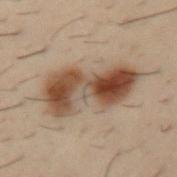follow-up: total-body-photography surveillance lesion; no biopsy | patient: male, aged approximately 30 | anatomic site: the left upper arm | automated metrics: a footprint of about 26 mm², an outline eccentricity of about 0.9 (0 = round, 1 = elongated), and a symmetry-axis asymmetry near 0.3 | imaging modality: ~15 mm tile from a whole-body skin photo | illumination: cross-polarized illumination.